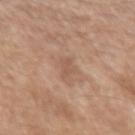notes: catalogued during a skin exam; not biopsied
illumination: white-light
image source: ~15 mm tile from a whole-body skin photo
size: ≈3 mm
site: the left upper arm
patient: male, aged 53 to 57
TBP lesion metrics: a footprint of about 3 mm² and an outline eccentricity of about 0.85 (0 = round, 1 = elongated)